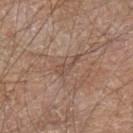– workup: total-body-photography surveillance lesion; no biopsy
– patient: male, aged 63–67
– TBP lesion metrics: an average lesion color of about L≈49 a*≈17 b*≈26 (CIELAB), a lesion–skin lightness drop of about 7, and a normalized lesion–skin contrast near 5; radial color variation of about 0
– image source: ~15 mm crop, total-body skin-cancer survey
– body site: the left forearm